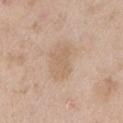{
  "biopsy_status": "not biopsied; imaged during a skin examination",
  "image": {
    "source": "total-body photography crop",
    "field_of_view_mm": 15
  },
  "lesion_size": {
    "long_diameter_mm_approx": 5.0
  },
  "patient": {
    "sex": "male",
    "age_approx": 50
  },
  "lighting": "white-light",
  "automated_metrics": {
    "area_mm2_approx": 11.0,
    "eccentricity": 0.85,
    "shape_asymmetry": 0.3,
    "cielab_L": 63,
    "cielab_a": 15,
    "cielab_b": 30,
    "vs_skin_darker_L": 6.0,
    "vs_skin_contrast_norm": 5.0,
    "lesion_detection_confidence_0_100": 100
  },
  "site": "left upper arm"
}